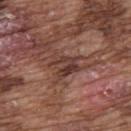biopsy_status: not biopsied; imaged during a skin examination